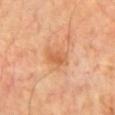Clinical impression:
Part of a total-body skin-imaging series; this lesion was reviewed on a skin check and was not flagged for biopsy.
Context:
A 15 mm close-up tile from a total-body photography series done for melanoma screening. A male patient, aged 63–67. On the chest.